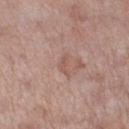{"biopsy_status": "not biopsied; imaged during a skin examination", "automated_metrics": {"area_mm2_approx": 2.5, "eccentricity": 0.85, "shape_asymmetry": 0.5, "vs_skin_darker_L": 7.0, "border_irregularity_0_10": 5.0, "peripheral_color_asymmetry": 0.0}, "site": "left lower leg", "lighting": "white-light", "lesion_size": {"long_diameter_mm_approx": 2.5}, "image": {"source": "total-body photography crop", "field_of_view_mm": 15}, "patient": {"sex": "female", "age_approx": 50}}